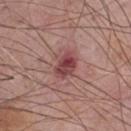Assessment:
Captured during whole-body skin photography for melanoma surveillance; the lesion was not biopsied.
Background:
The lesion-visualizer software estimated a lesion area of about 7.5 mm² and an eccentricity of roughly 0.7. The software also gave a mean CIELAB color near L≈45 a*≈26 b*≈20, about 12 CIELAB-L* units darker than the surrounding skin, and a lesion-to-skin contrast of about 9 (normalized; higher = more distinct). The software also gave border irregularity of about 3 on a 0–10 scale, internal color variation of about 7 on a 0–10 scale, and a peripheral color-asymmetry measure near 2.5. The analysis additionally found an automated nevus-likeness rating near 5 out of 100. A 15 mm close-up tile from a total-body photography series done for melanoma screening. A male patient roughly 75 years of age. Longest diameter approximately 3.5 mm. On the chest.